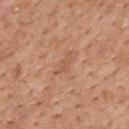Findings:
– workup — imaged on a skin check; not biopsied
– diameter — ~3 mm (longest diameter)
– imaging modality — total-body-photography crop, ~15 mm field of view
– anatomic site — the mid back
– patient — male, approximately 60 years of age